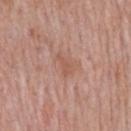{
  "biopsy_status": "not biopsied; imaged during a skin examination",
  "patient": {
    "sex": "male",
    "age_approx": 55
  },
  "lesion_size": {
    "long_diameter_mm_approx": 2.5
  },
  "automated_metrics": {
    "cielab_L": 55,
    "cielab_a": 22,
    "cielab_b": 29,
    "vs_skin_darker_L": 7.0,
    "vs_skin_contrast_norm": 5.5,
    "color_variation_0_10": 0.5,
    "peripheral_color_asymmetry": 0.0,
    "lesion_detection_confidence_0_100": 100
  },
  "image": {
    "source": "total-body photography crop",
    "field_of_view_mm": 15
  },
  "site": "mid back",
  "lighting": "white-light"
}